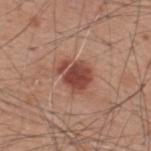The lesion was tiled from a total-body skin photograph and was not biopsied. A male patient approximately 60 years of age. The tile uses white-light illumination. The lesion's longest dimension is about 4 mm. From the upper back. A lesion tile, about 15 mm wide, cut from a 3D total-body photograph.